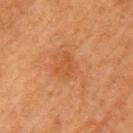Clinical impression: The lesion was tiled from a total-body skin photograph and was not biopsied. Acquisition and patient details: A female subject in their mid-50s. Imaged with cross-polarized lighting. Automated tile analysis of the lesion measured a shape eccentricity near 0.65 and a symmetry-axis asymmetry near 0.4. The software also gave a border-irregularity rating of about 4/10, internal color variation of about 2 on a 0–10 scale, and a peripheral color-asymmetry measure near 0.5. And it measured a classifier nevus-likeness of about 0/100. The lesion is located on the left upper arm. A 15 mm close-up extracted from a 3D total-body photography capture.